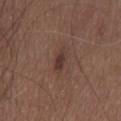Findings:
– follow-up — total-body-photography surveillance lesion; no biopsy
– size — ~2.5 mm (longest diameter)
– anatomic site — the head or neck
– acquisition — ~15 mm crop, total-body skin-cancer survey
– patient — female, aged around 65
– automated lesion analysis — a lesion area of about 3 mm², an eccentricity of roughly 0.85, and a symmetry-axis asymmetry near 0.3; a classifier nevus-likeness of about 70/100 and a detector confidence of about 100 out of 100 that the crop contains a lesion
– tile lighting — white-light illumination